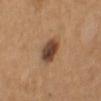notes: total-body-photography surveillance lesion; no biopsy
location: the left upper arm
subject: female, in their 40s
imaging modality: ~15 mm crop, total-body skin-cancer survey
tile lighting: white-light illumination